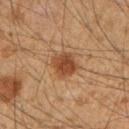The lesion was tiled from a total-body skin photograph and was not biopsied.
A 15 mm close-up tile from a total-body photography series done for melanoma screening.
A male patient aged around 50.
Located on the arm.